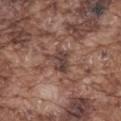Recorded during total-body skin imaging; not selected for excision or biopsy.